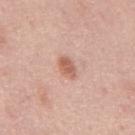The lesion was photographed on a routine skin check and not biopsied; there is no pathology result. The lesion is located on the mid back. The subject is a male roughly 45 years of age. About 3 mm across. A region of skin cropped from a whole-body photographic capture, roughly 15 mm wide. Imaged with white-light lighting. Automated tile analysis of the lesion measured an average lesion color of about L≈61 a*≈23 b*≈30 (CIELAB), about 11 CIELAB-L* units darker than the surrounding skin, and a normalized lesion–skin contrast near 7.5. It also reported a border-irregularity index near 1.5/10 and a within-lesion color-variation index near 2/10. The software also gave a classifier nevus-likeness of about 90/100 and a detector confidence of about 100 out of 100 that the crop contains a lesion.